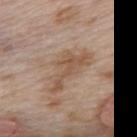Q: Was this lesion biopsied?
A: catalogued during a skin exam; not biopsied
Q: Where on the body is the lesion?
A: the upper back
Q: What lighting was used for the tile?
A: white-light illumination
Q: What kind of image is this?
A: total-body-photography crop, ~15 mm field of view
Q: What is the lesion's diameter?
A: ~6 mm (longest diameter)
Q: Who is the patient?
A: female, in their mid-60s